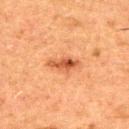Case summary:
– lighting · cross-polarized illumination
– site · the upper back
– patient · male, approximately 50 years of age
– acquisition · ~15 mm tile from a whole-body skin photo